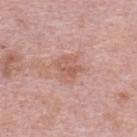Assessment:
Captured during whole-body skin photography for melanoma surveillance; the lesion was not biopsied.
Image and clinical context:
A female patient, in their mid-60s. From the upper back. The recorded lesion diameter is about 3 mm. A 15 mm crop from a total-body photograph taken for skin-cancer surveillance.